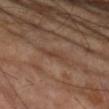Recorded during total-body skin imaging; not selected for excision or biopsy. On the left upper arm. A 15 mm crop from a total-body photograph taken for skin-cancer surveillance. A male subject about 60 years old. About 3 mm across.